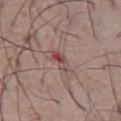Q: Was a biopsy performed?
A: no biopsy performed (imaged during a skin exam)
Q: How was the tile lit?
A: white-light
Q: What are the patient's age and sex?
A: male, aged 73 to 77
Q: Lesion location?
A: the chest
Q: What is the imaging modality?
A: 15 mm crop, total-body photography